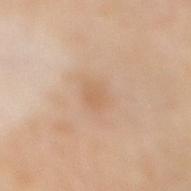Clinical summary:
The tile uses cross-polarized illumination. Approximately 2.5 mm at its widest. Located on the right lower leg. The lesion-visualizer software estimated a lesion color around L≈64 a*≈18 b*≈34 in CIELAB, about 6 CIELAB-L* units darker than the surrounding skin, and a lesion-to-skin contrast of about 4.5 (normalized; higher = more distinct). The software also gave an automated nevus-likeness rating near 0 out of 100. A male patient, aged approximately 55. Cropped from a total-body skin-imaging series; the visible field is about 15 mm.